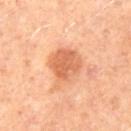Assessment: Recorded during total-body skin imaging; not selected for excision or biopsy.